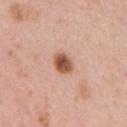Part of a total-body skin-imaging series; this lesion was reviewed on a skin check and was not flagged for biopsy. The lesion is located on the right upper arm. A 15 mm close-up tile from a total-body photography series done for melanoma screening. Automated tile analysis of the lesion measured internal color variation of about 4.5 on a 0–10 scale and peripheral color asymmetry of about 1.5. A female patient, aged 33–37. This is a white-light tile.